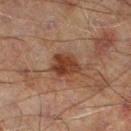Impression: Recorded during total-body skin imaging; not selected for excision or biopsy. Acquisition and patient details: Automated tile analysis of the lesion measured an average lesion color of about L≈28 a*≈18 b*≈24 (CIELAB), roughly 9 lightness units darker than nearby skin, and a normalized lesion–skin contrast near 9.5. The software also gave border irregularity of about 2.5 on a 0–10 scale and a within-lesion color-variation index near 3/10. And it measured a nevus-likeness score of about 70/100 and a lesion-detection confidence of about 100/100. This is a cross-polarized tile. The lesion is on the left lower leg. A male patient roughly 60 years of age. Cropped from a whole-body photographic skin survey; the tile spans about 15 mm. Longest diameter approximately 3.5 mm.